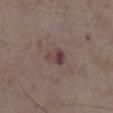The lesion was tiled from a total-body skin photograph and was not biopsied.